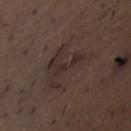Q: Was a biopsy performed?
A: no biopsy performed (imaged during a skin exam)
Q: What is the lesion's diameter?
A: ≈7 mm
Q: What are the patient's age and sex?
A: male, aged 48 to 52
Q: How was this image acquired?
A: 15 mm crop, total-body photography
Q: What lighting was used for the tile?
A: white-light illumination
Q: What is the anatomic site?
A: the chest